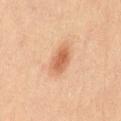A roughly 15 mm field-of-view crop from a total-body skin photograph. The patient is a female in their 20s. This is a cross-polarized tile. Measured at roughly 3.5 mm in maximum diameter. Located on the left thigh. The lesion-visualizer software estimated a lesion area of about 7 mm² and a symmetry-axis asymmetry near 0.2. It also reported roughly 10 lightness units darker than nearby skin. And it measured border irregularity of about 2 on a 0–10 scale, a within-lesion color-variation index near 2.5/10, and radial color variation of about 1.